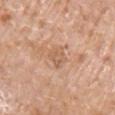Clinical impression:
Captured during whole-body skin photography for melanoma surveillance; the lesion was not biopsied.
Acquisition and patient details:
About 3 mm across. The lesion-visualizer software estimated a footprint of about 5 mm² and an eccentricity of roughly 0.5. The lesion is located on the arm. Imaged with white-light lighting. Cropped from a total-body skin-imaging series; the visible field is about 15 mm. The patient is a female roughly 85 years of age or older.